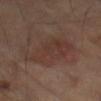Case summary:
- notes — catalogued during a skin exam; not biopsied
- acquisition — total-body-photography crop, ~15 mm field of view
- patient — male, in their 70s
- TBP lesion metrics — a lesion color around L≈34 a*≈17 b*≈22 in CIELAB, about 5 CIELAB-L* units darker than the surrounding skin, and a normalized lesion–skin contrast near 5.5; lesion-presence confidence of about 100/100
- tile lighting — cross-polarized
- anatomic site — the left thigh